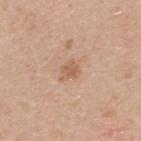Q: Was this lesion biopsied?
A: total-body-photography surveillance lesion; no biopsy
Q: What did automated image analysis measure?
A: a mean CIELAB color near L≈59 a*≈21 b*≈33 and a lesion–skin lightness drop of about 8
Q: How was this image acquired?
A: 15 mm crop, total-body photography
Q: Patient demographics?
A: male, in their mid- to late 60s
Q: What is the lesion's diameter?
A: ~2.5 mm (longest diameter)
Q: Illumination type?
A: white-light
Q: Where on the body is the lesion?
A: the upper back